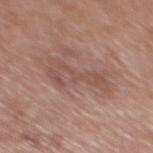Recorded during total-body skin imaging; not selected for excision or biopsy.
A close-up tile cropped from a whole-body skin photograph, about 15 mm across.
The tile uses white-light illumination.
Automated tile analysis of the lesion measured roughly 7 lightness units darker than nearby skin and a lesion-to-skin contrast of about 5.5 (normalized; higher = more distinct). It also reported internal color variation of about 2.5 on a 0–10 scale and radial color variation of about 0.5. It also reported a nevus-likeness score of about 0/100 and lesion-presence confidence of about 100/100.
From the mid back.
A male subject in their 70s.
About 6 mm across.